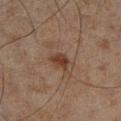Q: Was a biopsy performed?
A: no biopsy performed (imaged during a skin exam)
Q: What lighting was used for the tile?
A: cross-polarized
Q: Patient demographics?
A: male, aged approximately 45
Q: What kind of image is this?
A: total-body-photography crop, ~15 mm field of view
Q: Lesion location?
A: the right lower leg